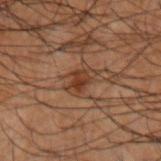workup — total-body-photography surveillance lesion; no biopsy | subject — male, aged 48–52 | site — the right forearm | lesion diameter — ~3.5 mm (longest diameter) | automated metrics — a symmetry-axis asymmetry near 0.25; a border-irregularity rating of about 3/10, a color-variation rating of about 4/10, and peripheral color asymmetry of about 1.5 | tile lighting — cross-polarized illumination | acquisition — ~15 mm crop, total-body skin-cancer survey.